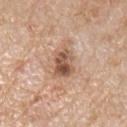workup — total-body-photography surveillance lesion; no biopsy | subject — male, aged approximately 60 | acquisition — 15 mm crop, total-body photography | illumination — white-light illumination | body site — the right upper arm.